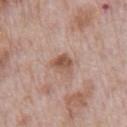Imaged during a routine full-body skin examination; the lesion was not biopsied and no histopathology is available. A 15 mm crop from a total-body photograph taken for skin-cancer surveillance. A male subject approximately 70 years of age. Approximately 3 mm at its widest. On the front of the torso. Captured under white-light illumination.